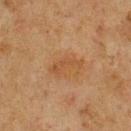Captured during whole-body skin photography for melanoma surveillance; the lesion was not biopsied. The subject is a male in their mid- to late 70s. The lesion is on the chest. A 15 mm close-up tile from a total-body photography series done for melanoma screening.